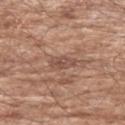Impression: This lesion was catalogued during total-body skin photography and was not selected for biopsy. Acquisition and patient details: Automated image analysis of the tile measured an eccentricity of roughly 0.8. The lesion is on the left upper arm. A 15 mm close-up extracted from a 3D total-body photography capture. The tile uses white-light illumination. The patient is a male aged approximately 65.